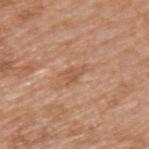Clinical impression:
Captured during whole-body skin photography for melanoma surveillance; the lesion was not biopsied.
Context:
A region of skin cropped from a whole-body photographic capture, roughly 15 mm wide. Located on the upper back. The patient is a male aged 63 to 67. Captured under white-light illumination. Automated tile analysis of the lesion measured a lesion color around L≈55 a*≈22 b*≈33 in CIELAB, roughly 7 lightness units darker than nearby skin, and a normalized lesion–skin contrast near 5.5. The software also gave a border-irregularity rating of about 4/10, a within-lesion color-variation index near 1.5/10, and a peripheral color-asymmetry measure near 0.5. And it measured an automated nevus-likeness rating near 0 out of 100. About 2.5 mm across.